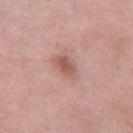Q: Was a biopsy performed?
A: no biopsy performed (imaged during a skin exam)
Q: Lesion size?
A: ~3.5 mm (longest diameter)
Q: Automated lesion metrics?
A: a border-irregularity rating of about 2/10 and peripheral color asymmetry of about 1; a classifier nevus-likeness of about 60/100 and a detector confidence of about 100 out of 100 that the crop contains a lesion
Q: What lighting was used for the tile?
A: white-light illumination
Q: How was this image acquired?
A: ~15 mm crop, total-body skin-cancer survey
Q: Lesion location?
A: the leg
Q: Who is the patient?
A: female, aged approximately 70